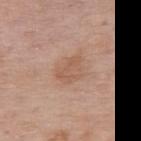Assessment:
Captured during whole-body skin photography for melanoma surveillance; the lesion was not biopsied.
Clinical summary:
The patient is a female roughly 50 years of age. The recorded lesion diameter is about 3.5 mm. The lesion-visualizer software estimated a classifier nevus-likeness of about 0/100 and a lesion-detection confidence of about 100/100. The tile uses white-light illumination. A close-up tile cropped from a whole-body skin photograph, about 15 mm across. The lesion is located on the upper back.